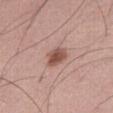<case>
  <biopsy_status>not biopsied; imaged during a skin examination</biopsy_status>
  <image>
    <source>total-body photography crop</source>
    <field_of_view_mm>15</field_of_view_mm>
  </image>
  <site>abdomen</site>
  <lesion_size>
    <long_diameter_mm_approx>3.0</long_diameter_mm_approx>
  </lesion_size>
  <patient>
    <sex>male</sex>
    <age_approx>55</age_approx>
  </patient>
  <automated_metrics>
    <border_irregularity_0_10>2.5</border_irregularity_0_10>
    <color_variation_0_10>3.0</color_variation_0_10>
    <peripheral_color_asymmetry>1.0</peripheral_color_asymmetry>
  </automated_metrics>
  <lighting>white-light</lighting>
</case>